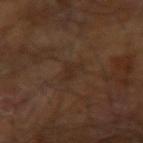{"biopsy_status": "not biopsied; imaged during a skin examination", "automated_metrics": {"cielab_L": 25, "cielab_a": 14, "cielab_b": 23, "vs_skin_darker_L": 4.0, "vs_skin_contrast_norm": 5.0, "border_irregularity_0_10": 4.5, "color_variation_0_10": 0.0, "peripheral_color_asymmetry": 0.0, "lesion_detection_confidence_0_100": 55}, "site": "right arm", "lighting": "cross-polarized", "patient": {"sex": "male", "age_approx": 60}, "image": {"source": "total-body photography crop", "field_of_view_mm": 15}}